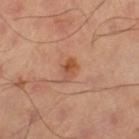The lesion was tiled from a total-body skin photograph and was not biopsied. This image is a 15 mm lesion crop taken from a total-body photograph. The lesion is on the left thigh. This is a cross-polarized tile. The lesion's longest dimension is about 3 mm.